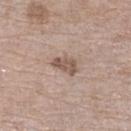biopsy_status: not biopsied; imaged during a skin examination
lighting: white-light
lesion_size:
  long_diameter_mm_approx: 3.5
site: left lower leg
patient:
  sex: female
  age_approx: 75
image:
  source: total-body photography crop
  field_of_view_mm: 15